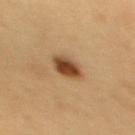Recorded during total-body skin imaging; not selected for excision or biopsy.
Imaged with cross-polarized lighting.
From the back.
The patient is a female in their 30s.
About 3 mm across.
A 15 mm close-up tile from a total-body photography series done for melanoma screening.